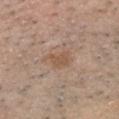The lesion was tiled from a total-body skin photograph and was not biopsied.
On the head or neck.
Approximately 3.5 mm at its widest.
A male subject aged 38 to 42.
A 15 mm close-up tile from a total-body photography series done for melanoma screening.
Captured under white-light illumination.